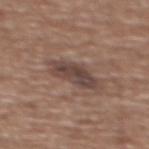Impression:
The lesion was tiled from a total-body skin photograph and was not biopsied.
Image and clinical context:
About 5 mm across. Captured under white-light illumination. On the upper back. A region of skin cropped from a whole-body photographic capture, roughly 15 mm wide. The subject is a male in their mid- to late 70s.